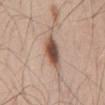<case>
  <biopsy_status>not biopsied; imaged during a skin examination</biopsy_status>
  <patient>
    <sex>male</sex>
    <age_approx>45</age_approx>
  </patient>
  <lesion_size>
    <long_diameter_mm_approx>4.0</long_diameter_mm_approx>
  </lesion_size>
  <site>lower back</site>
  <lighting>white-light</lighting>
  <image>
    <source>total-body photography crop</source>
    <field_of_view_mm>15</field_of_view_mm>
  </image>
</case>